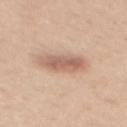This lesion was catalogued during total-body skin photography and was not selected for biopsy. The lesion is located on the mid back. Captured under white-light illumination. The subject is a female in their mid-40s. A roughly 15 mm field-of-view crop from a total-body skin photograph. About 4.5 mm across.